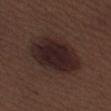workup: imaged on a skin check; not biopsied | tile lighting: white-light illumination | image: ~15 mm crop, total-body skin-cancer survey | subject: male, aged around 70 | lesion size: ≈7.5 mm | location: the left thigh | image-analysis metrics: a lesion area of about 26 mm² and a shape-asymmetry score of about 0.1 (0 = symmetric); about 9 CIELAB-L* units darker than the surrounding skin and a lesion-to-skin contrast of about 12 (normalized; higher = more distinct); a classifier nevus-likeness of about 75/100.